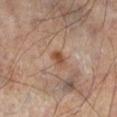Impression:
The lesion was tiled from a total-body skin photograph and was not biopsied.
Acquisition and patient details:
Measured at roughly 2 mm in maximum diameter. A male patient approximately 65 years of age. On the left lower leg. Cropped from a whole-body photographic skin survey; the tile spans about 15 mm.